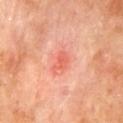Part of a total-body skin-imaging series; this lesion was reviewed on a skin check and was not flagged for biopsy.
A male subject, aged 68 to 72.
On the back.
A roughly 15 mm field-of-view crop from a total-body skin photograph.